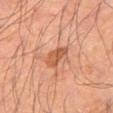{"patient": {"sex": "male", "age_approx": 55}, "site": "right thigh", "lighting": "cross-polarized", "image": {"source": "total-body photography crop", "field_of_view_mm": 15}, "lesion_size": {"long_diameter_mm_approx": 3.0}}